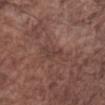No biopsy was performed on this lesion — it was imaged during a full skin examination and was not determined to be concerning. The lesion is on the right forearm. Measured at roughly 2.5 mm in maximum diameter. The lesion-visualizer software estimated a border-irregularity rating of about 6/10, internal color variation of about 0 on a 0–10 scale, and radial color variation of about 0. It also reported a nevus-likeness score of about 0/100. Imaged with white-light lighting. A region of skin cropped from a whole-body photographic capture, roughly 15 mm wide. A male patient, aged around 75.